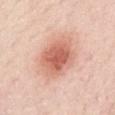Imaged during a routine full-body skin examination; the lesion was not biopsied and no histopathology is available.
From the chest.
A female subject, approximately 40 years of age.
Imaged with white-light lighting.
A close-up tile cropped from a whole-body skin photograph, about 15 mm across.
About 5 mm across.